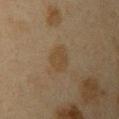Q: Was this lesion biopsied?
A: no biopsy performed (imaged during a skin exam)
Q: What lighting was used for the tile?
A: cross-polarized illumination
Q: Lesion size?
A: ~3.5 mm (longest diameter)
Q: Patient demographics?
A: female, aged 38 to 42
Q: Automated lesion metrics?
A: an area of roughly 6.5 mm², an eccentricity of roughly 0.7, and a shape-asymmetry score of about 0.2 (0 = symmetric); a lesion color around L≈36 a*≈11 b*≈27 in CIELAB, roughly 5 lightness units darker than nearby skin, and a normalized lesion–skin contrast near 6; a border-irregularity index near 2/10 and radial color variation of about 0.5
Q: Where on the body is the lesion?
A: the left upper arm
Q: How was this image acquired?
A: total-body-photography crop, ~15 mm field of view